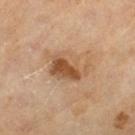The lesion was tiled from a total-body skin photograph and was not biopsied.
Measured at roughly 5.5 mm in maximum diameter.
Imaged with cross-polarized lighting.
A roughly 15 mm field-of-view crop from a total-body skin photograph.
The lesion-visualizer software estimated a nevus-likeness score of about 70/100.
The subject is a male in their mid- to late 60s.